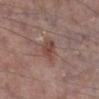The lesion was photographed on a routine skin check and not biopsied; there is no pathology result. The total-body-photography lesion software estimated an outline eccentricity of about 0.6 (0 = round, 1 = elongated). It also reported a lesion–skin lightness drop of about 9. The analysis additionally found an automated nevus-likeness rating near 5 out of 100 and a lesion-detection confidence of about 100/100. From the right lower leg. Imaged with white-light lighting. A 15 mm close-up tile from a total-body photography series done for melanoma screening. About 2.5 mm across. A male patient about 65 years old.